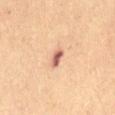Imaged during a routine full-body skin examination; the lesion was not biopsied and no histopathology is available. Longest diameter approximately 2.5 mm. The subject is a female in their 70s. This image is a 15 mm lesion crop taken from a total-body photograph. Located on the abdomen. Captured under cross-polarized illumination.